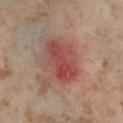Imaged during a routine full-body skin examination; the lesion was not biopsied and no histopathology is available. Cropped from a whole-body photographic skin survey; the tile spans about 15 mm. A female subject aged 53–57. This is a cross-polarized tile. The lesion is on the left thigh.